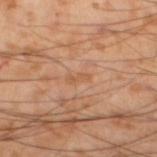This lesion was catalogued during total-body skin photography and was not selected for biopsy. A male subject, in their mid- to late 50s. From the left lower leg. A close-up tile cropped from a whole-body skin photograph, about 15 mm across.